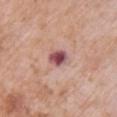* lesion size: ~2.5 mm (longest diameter)
* automated metrics: a lesion area of about 4 mm² and a shape eccentricity near 0.65; a border-irregularity rating of about 2.5/10, internal color variation of about 6 on a 0–10 scale, and peripheral color asymmetry of about 2; a nevus-likeness score of about 25/100 and a detector confidence of about 100 out of 100 that the crop contains a lesion
* subject: female, about 70 years old
* image source: ~15 mm crop, total-body skin-cancer survey
* body site: the chest
* tile lighting: white-light illumination